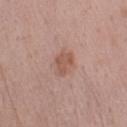Captured during whole-body skin photography for melanoma surveillance; the lesion was not biopsied. A male subject, aged 53 to 57. On the left upper arm. This image is a 15 mm lesion crop taken from a total-body photograph.